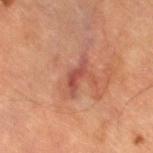Captured during whole-body skin photography for melanoma surveillance; the lesion was not biopsied.
Approximately 4 mm at its widest.
A 15 mm crop from a total-body photograph taken for skin-cancer surveillance.
From the left lower leg.
A male patient, aged around 85 or older.